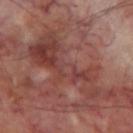follow-up = no biopsy performed (imaged during a skin exam)
acquisition = total-body-photography crop, ~15 mm field of view
patient = male, roughly 70 years of age
location = the leg